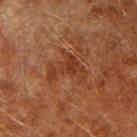<record>
  <biopsy_status>not biopsied; imaged during a skin examination</biopsy_status>
</record>